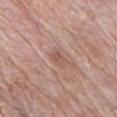The lesion was photographed on a routine skin check and not biopsied; there is no pathology result. A close-up tile cropped from a whole-body skin photograph, about 15 mm across. Located on the chest. Approximately 2.5 mm at its widest. Imaged with white-light lighting. The subject is a male aged 73 to 77.